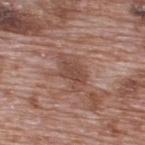The lesion was photographed on a routine skin check and not biopsied; there is no pathology result. Located on the upper back. Cropped from a total-body skin-imaging series; the visible field is about 15 mm. A male patient about 70 years old.